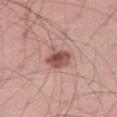No biopsy was performed on this lesion — it was imaged during a full skin examination and was not determined to be concerning.
A male patient aged around 45.
Cropped from a total-body skin-imaging series; the visible field is about 15 mm.
The recorded lesion diameter is about 3 mm.
An algorithmic analysis of the crop reported a lesion color around L≈52 a*≈23 b*≈26 in CIELAB, roughly 13 lightness units darker than nearby skin, and a normalized lesion–skin contrast near 9. The analysis additionally found a nevus-likeness score of about 85/100 and lesion-presence confidence of about 100/100.
On the left thigh.
The tile uses white-light illumination.